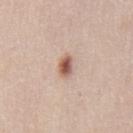– biopsy status — imaged on a skin check; not biopsied
– image-analysis metrics — an area of roughly 3.5 mm²; a lesion color around L≈57 a*≈21 b*≈28 in CIELAB, a lesion–skin lightness drop of about 16, and a normalized border contrast of about 10
– size — ~2.5 mm (longest diameter)
– imaging modality — ~15 mm tile from a whole-body skin photo
– patient — male, about 45 years old
– location — the chest